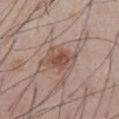{"biopsy_status": "not biopsied; imaged during a skin examination", "image": {"source": "total-body photography crop", "field_of_view_mm": 15}, "lighting": "white-light", "site": "front of the torso", "patient": {"sex": "male", "age_approx": 55}}